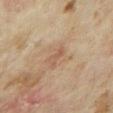  biopsy_status: not biopsied; imaged during a skin examination
  site: right forearm
  patient:
    sex: female
    age_approx: 35
  image:
    source: total-body photography crop
    field_of_view_mm: 15
  lesion_size:
    long_diameter_mm_approx: 3.0
  lighting: cross-polarized